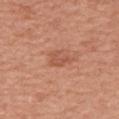| feature | finding |
|---|---|
| subject | female, approximately 60 years of age |
| lesion diameter | about 2.5 mm |
| acquisition | ~15 mm crop, total-body skin-cancer survey |
| body site | the right upper arm |